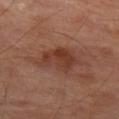Captured during whole-body skin photography for melanoma surveillance; the lesion was not biopsied. Approximately 4.5 mm at its widest. A region of skin cropped from a whole-body photographic capture, roughly 15 mm wide. An algorithmic analysis of the crop reported a color-variation rating of about 4/10 and peripheral color asymmetry of about 1.5. And it measured an automated nevus-likeness rating near 20 out of 100. The tile uses cross-polarized illumination. A male patient, roughly 70 years of age. Located on the left thigh.